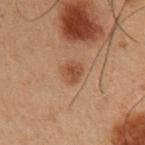The lesion was tiled from a total-body skin photograph and was not biopsied.
The patient is a male aged approximately 55.
The lesion is on the right upper arm.
Approximately 2.5 mm at its widest.
A region of skin cropped from a whole-body photographic capture, roughly 15 mm wide.
Captured under cross-polarized illumination.